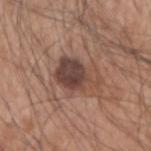Assessment: The lesion was tiled from a total-body skin photograph and was not biopsied. Context: The tile uses white-light illumination. Approximately 5 mm at its widest. Automated image analysis of the tile measured a shape eccentricity near 0.75 and a symmetry-axis asymmetry near 0.4. It also reported a lesion–skin lightness drop of about 12 and a lesion-to-skin contrast of about 9.5 (normalized; higher = more distinct). It also reported a border-irregularity index near 4.5/10, a color-variation rating of about 4.5/10, and peripheral color asymmetry of about 1.5. It also reported a classifier nevus-likeness of about 40/100. From the right forearm. The patient is a male aged around 55. A 15 mm crop from a total-body photograph taken for skin-cancer surveillance.